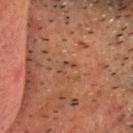notes = no biopsy performed (imaged during a skin exam); body site = the head or neck; lesion size = ≈2.5 mm; lighting = cross-polarized illumination; image source = total-body-photography crop, ~15 mm field of view; patient = male, about 50 years old; automated metrics = roughly 5 lightness units darker than nearby skin.